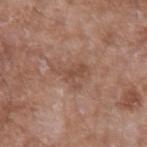  biopsy_status: not biopsied; imaged during a skin examination
  site: left forearm
  image:
    source: total-body photography crop
    field_of_view_mm: 15
  patient:
    sex: male
    age_approx: 60